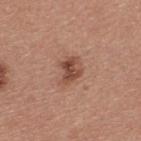Impression: The lesion was tiled from a total-body skin photograph and was not biopsied. Clinical summary: A male patient, approximately 40 years of age. Located on the upper back. A lesion tile, about 15 mm wide, cut from a 3D total-body photograph.